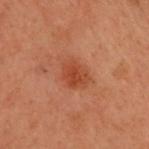notes: catalogued during a skin exam; not biopsied
automated metrics: an area of roughly 7 mm², a shape eccentricity near 0.7, and a symmetry-axis asymmetry near 0.25; a color-variation rating of about 2.5/10 and radial color variation of about 1
lesion size: about 3.5 mm
image source: ~15 mm tile from a whole-body skin photo
subject: male, approximately 55 years of age
lighting: cross-polarized illumination
anatomic site: the head or neck